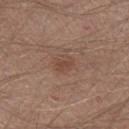<lesion>
  <biopsy_status>not biopsied; imaged during a skin examination</biopsy_status>
  <lighting>white-light</lighting>
  <site>left forearm</site>
  <lesion_size>
    <long_diameter_mm_approx>2.5</long_diameter_mm_approx>
  </lesion_size>
  <patient>
    <sex>male</sex>
    <age_approx>40</age_approx>
  </patient>
  <image>
    <source>total-body photography crop</source>
    <field_of_view_mm>15</field_of_view_mm>
  </image>
</lesion>